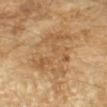biopsy status=no biopsy performed (imaged during a skin exam) | site=the left forearm | lesion size=about 7.5 mm | patient=female, aged 68–72 | tile lighting=cross-polarized illumination | image source=total-body-photography crop, ~15 mm field of view.